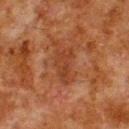Recorded during total-body skin imaging; not selected for excision or biopsy.
The lesion-visualizer software estimated an area of roughly 6.5 mm². It also reported a classifier nevus-likeness of about 0/100 and lesion-presence confidence of about 100/100.
On the upper back.
A lesion tile, about 15 mm wide, cut from a 3D total-body photograph.
The tile uses cross-polarized illumination.
The subject is a male roughly 80 years of age.